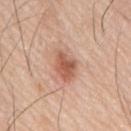Findings:
- biopsy status — catalogued during a skin exam; not biopsied
- tile lighting — white-light
- automated metrics — a mean CIELAB color near L≈58 a*≈24 b*≈31, about 12 CIELAB-L* units darker than the surrounding skin, and a normalized lesion–skin contrast near 8
- patient — male, aged 78 to 82
- location — the mid back
- image — 15 mm crop, total-body photography